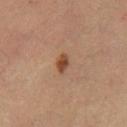Imaged during a routine full-body skin examination; the lesion was not biopsied and no histopathology is available. A 15 mm close-up tile from a total-body photography series done for melanoma screening. The lesion is located on the right thigh. A female patient, in their mid- to late 40s.